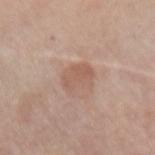Q: Was a biopsy performed?
A: catalogued during a skin exam; not biopsied
Q: Where on the body is the lesion?
A: the left forearm
Q: What is the imaging modality?
A: ~15 mm tile from a whole-body skin photo
Q: Patient demographics?
A: male, aged 53–57